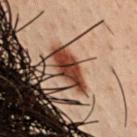The lesion was tiled from a total-body skin photograph and was not biopsied.
This image is a 15 mm lesion crop taken from a total-body photograph.
The lesion is on the front of the torso.
About 3 mm across.
A male patient aged 28 to 32.
This is a cross-polarized tile.